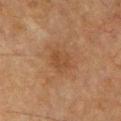Recorded during total-body skin imaging; not selected for excision or biopsy. Captured under cross-polarized illumination. On the front of the torso. Longest diameter approximately 3 mm. A male subject aged around 65. An algorithmic analysis of the crop reported a footprint of about 6 mm², a shape eccentricity near 0.65, and a shape-asymmetry score of about 0.25 (0 = symmetric). It also reported a border-irregularity rating of about 2.5/10 and a within-lesion color-variation index near 2/10. Cropped from a total-body skin-imaging series; the visible field is about 15 mm.